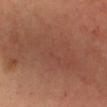- workup: catalogued during a skin exam; not biopsied
- location: the chest
- lesion diameter: ≈13 mm
- automated lesion analysis: a footprint of about 60 mm², an eccentricity of roughly 0.9, and two-axis asymmetry of about 0.35; a color-variation rating of about 3/10 and a peripheral color-asymmetry measure near 1
- image: 15 mm crop, total-body photography
- patient: female, aged 38 to 42
- tile lighting: cross-polarized